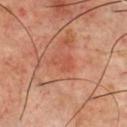<lesion>
<biopsy_status>not biopsied; imaged during a skin examination</biopsy_status>
<patient>
  <sex>male</sex>
  <age_approx>70</age_approx>
</patient>
<site>chest</site>
<image>
  <source>total-body photography crop</source>
  <field_of_view_mm>15</field_of_view_mm>
</image>
</lesion>